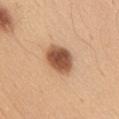  biopsy_status: not biopsied; imaged during a skin examination
  lighting: white-light
  lesion_size:
    long_diameter_mm_approx: 4.5
  site: front of the torso
  image:
    source: total-body photography crop
    field_of_view_mm: 15
  patient:
    sex: male
    age_approx: 50
  automated_metrics:
    area_mm2_approx: 10.0
    eccentricity: 0.7
    shape_asymmetry: 0.15
    cielab_L: 53
    cielab_a: 22
    cielab_b: 34
    vs_skin_darker_L: 18.0
    vs_skin_contrast_norm: 11.5